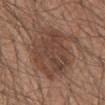Imaged during a routine full-body skin examination; the lesion was not biopsied and no histopathology is available. On the abdomen. This is a white-light tile. The subject is a male in their mid-60s. Measured at roughly 8 mm in maximum diameter. Automated image analysis of the tile measured a lesion color around L≈43 a*≈18 b*≈26 in CIELAB, roughly 9 lightness units darker than nearby skin, and a normalized border contrast of about 7. A 15 mm crop from a total-body photograph taken for skin-cancer surveillance.